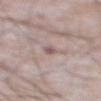The total-body-photography lesion software estimated a lesion area of about 2.5 mm², a shape eccentricity near 0.9, and a symmetry-axis asymmetry near 0.45. Longest diameter approximately 2.5 mm. A male subject in their mid- to late 60s. Cropped from a whole-body photographic skin survey; the tile spans about 15 mm. This is a white-light tile. From the abdomen.